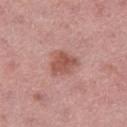workup: no biopsy performed (imaged during a skin exam) | diameter: ≈3.5 mm | automated lesion analysis: a border-irregularity index near 3/10, a color-variation rating of about 3/10, and a peripheral color-asymmetry measure near 1; a nevus-likeness score of about 55/100 and a lesion-detection confidence of about 100/100 | acquisition: 15 mm crop, total-body photography | patient: female, roughly 45 years of age | body site: the right thigh.